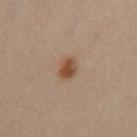Notes:
– site: the left leg
– image source: total-body-photography crop, ~15 mm field of view
– size: about 3 mm
– subject: female, approximately 40 years of age
– image-analysis metrics: a border-irregularity index near 1.5/10, a color-variation rating of about 3.5/10, and peripheral color asymmetry of about 1
– illumination: cross-polarized